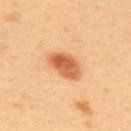follow-up: total-body-photography surveillance lesion; no biopsy
imaging modality: total-body-photography crop, ~15 mm field of view
image-analysis metrics: a lesion area of about 9.5 mm², an outline eccentricity of about 0.8 (0 = round, 1 = elongated), and two-axis asymmetry of about 0.2; about 15 CIELAB-L* units darker than the surrounding skin and a normalized border contrast of about 9; a border-irregularity index near 2/10, a color-variation rating of about 5/10, and a peripheral color-asymmetry measure near 1.5; a classifier nevus-likeness of about 100/100 and a lesion-detection confidence of about 100/100
lighting: cross-polarized
body site: the upper back
lesion diameter: ~4.5 mm (longest diameter)
subject: male, aged around 40